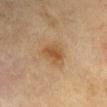This lesion was catalogued during total-body skin photography and was not selected for biopsy.
From the left upper arm.
The patient is a male in their 70s.
Cropped from a total-body skin-imaging series; the visible field is about 15 mm.